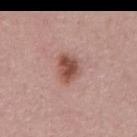biopsy status — no biopsy performed (imaged during a skin exam)
tile lighting — white-light
diameter — ≈3.5 mm
image-analysis metrics — a shape eccentricity near 0.7 and a symmetry-axis asymmetry near 0.3; a mean CIELAB color near L≈50 a*≈24 b*≈26, roughly 13 lightness units darker than nearby skin, and a lesion-to-skin contrast of about 9.5 (normalized; higher = more distinct); a within-lesion color-variation index near 4/10 and radial color variation of about 1.5; a classifier nevus-likeness of about 95/100 and a detector confidence of about 100 out of 100 that the crop contains a lesion
image source — ~15 mm crop, total-body skin-cancer survey
subject — male, in their 60s
site — the abdomen